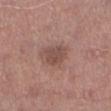Assessment: Captured during whole-body skin photography for melanoma surveillance; the lesion was not biopsied. Image and clinical context: Longest diameter approximately 3.5 mm. From the left lower leg. A 15 mm crop from a total-body photograph taken for skin-cancer surveillance. A male subject, aged 63–67. Imaged with white-light lighting.